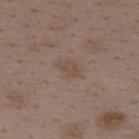Impression:
Recorded during total-body skin imaging; not selected for excision or biopsy.
Acquisition and patient details:
A 15 mm close-up extracted from a 3D total-body photography capture. A female patient, aged approximately 35. From the back.